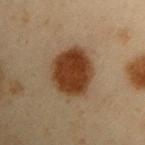Assessment:
Imaged during a routine full-body skin examination; the lesion was not biopsied and no histopathology is available.
Image and clinical context:
The lesion is on the left upper arm. The patient is a male aged approximately 55. Automated tile analysis of the lesion measured a footprint of about 19 mm², an eccentricity of roughly 0.55, and two-axis asymmetry of about 0.15. The software also gave an average lesion color of about L≈30 a*≈17 b*≈28 (CIELAB), a lesion–skin lightness drop of about 14, and a normalized border contrast of about 13.5. The software also gave a nevus-likeness score of about 100/100 and a detector confidence of about 100 out of 100 that the crop contains a lesion. A lesion tile, about 15 mm wide, cut from a 3D total-body photograph. The recorded lesion diameter is about 5.5 mm.